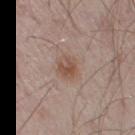<case>
<biopsy_status>not biopsied; imaged during a skin examination</biopsy_status>
<lighting>white-light</lighting>
<image>
  <source>total-body photography crop</source>
  <field_of_view_mm>15</field_of_view_mm>
</image>
<automated_metrics>
  <eccentricity>0.65</eccentricity>
  <shape_asymmetry>0.2</shape_asymmetry>
  <nevus_likeness_0_100>90</nevus_likeness_0_100>
</automated_metrics>
<site>right upper arm</site>
<patient>
  <sex>male</sex>
  <age_approx>65</age_approx>
</patient>
</case>